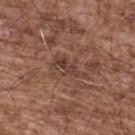biopsy_status: not biopsied; imaged during a skin examination
image:
  source: total-body photography crop
  field_of_view_mm: 15
patient:
  sex: male
  age_approx: 55
site: upper back